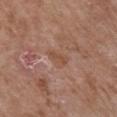Imaged during a routine full-body skin examination; the lesion was not biopsied and no histopathology is available. A 15 mm close-up extracted from a 3D total-body photography capture. This is a white-light tile. The subject is a female aged approximately 80. Automated image analysis of the tile measured a lesion color around L≈50 a*≈20 b*≈29 in CIELAB, a lesion–skin lightness drop of about 6, and a normalized border contrast of about 5.5. It also reported a border-irregularity rating of about 3/10 and peripheral color asymmetry of about 0.5. It also reported an automated nevus-likeness rating near 0 out of 100 and a lesion-detection confidence of about 100/100. Approximately 3 mm at its widest. Located on the chest.